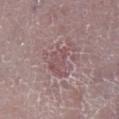Notes:
• workup · catalogued during a skin exam; not biopsied
• lesion diameter · ≈4.5 mm
• location · the right lower leg
• imaging modality · 15 mm crop, total-body photography
• patient · female, aged 68–72
• tile lighting · white-light